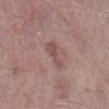Recorded during total-body skin imaging; not selected for excision or biopsy. The recorded lesion diameter is about 3.5 mm. A male subject approximately 55 years of age. Captured under white-light illumination. A roughly 15 mm field-of-view crop from a total-body skin photograph. The lesion is on the right lower leg.